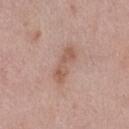Notes:
- body site — the leg
- lighting — white-light
- acquisition — ~15 mm crop, total-body skin-cancer survey
- subject — female, aged approximately 40
- lesion size — about 4.5 mm
- TBP lesion metrics — a footprint of about 7 mm² and a shape eccentricity near 0.95; a nevus-likeness score of about 0/100